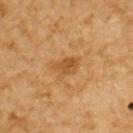Clinical impression:
Part of a total-body skin-imaging series; this lesion was reviewed on a skin check and was not flagged for biopsy.
Image and clinical context:
A male patient in their mid-80s or older. A 15 mm close-up extracted from a 3D total-body photography capture. The lesion is on the upper back. Measured at roughly 3 mm in maximum diameter.